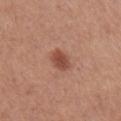Assessment:
Recorded during total-body skin imaging; not selected for excision or biopsy.
Context:
A close-up tile cropped from a whole-body skin photograph, about 15 mm across. A female patient in their mid-40s. Automated tile analysis of the lesion measured about 11 CIELAB-L* units darker than the surrounding skin and a normalized lesion–skin contrast near 8.5. It also reported an automated nevus-likeness rating near 95 out of 100 and a detector confidence of about 100 out of 100 that the crop contains a lesion. Approximately 2.5 mm at its widest. The lesion is on the arm.